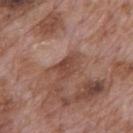Q: Is there a histopathology result?
A: total-body-photography surveillance lesion; no biopsy
Q: What is the imaging modality?
A: ~15 mm crop, total-body skin-cancer survey
Q: Patient demographics?
A: male, about 70 years old
Q: What lighting was used for the tile?
A: white-light illumination
Q: What is the anatomic site?
A: the mid back